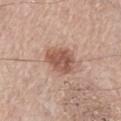Captured during whole-body skin photography for melanoma surveillance; the lesion was not biopsied.
From the leg.
This image is a 15 mm lesion crop taken from a total-body photograph.
Automated tile analysis of the lesion measured a footprint of about 9 mm², a shape eccentricity near 0.65, and a symmetry-axis asymmetry near 0.3. The analysis additionally found an average lesion color of about L≈54 a*≈21 b*≈27 (CIELAB). The software also gave a color-variation rating of about 3.5/10 and peripheral color asymmetry of about 1.
Captured under white-light illumination.
A male subject about 75 years old.